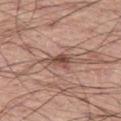| feature | finding |
|---|---|
| workup | imaged on a skin check; not biopsied |
| patient | male, roughly 60 years of age |
| body site | the left thigh |
| diameter | ~3 mm (longest diameter) |
| image source | ~15 mm tile from a whole-body skin photo |
| image-analysis metrics | a lesion area of about 4.5 mm², an outline eccentricity of about 0.85 (0 = round, 1 = elongated), and a shape-asymmetry score of about 0.4 (0 = symmetric); roughly 12 lightness units darker than nearby skin and a normalized border contrast of about 8.5; a classifier nevus-likeness of about 50/100 and lesion-presence confidence of about 100/100 |
| lighting | white-light illumination |